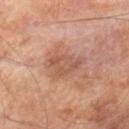workup — imaged on a skin check; not biopsied | acquisition — 15 mm crop, total-body photography | patient — male, in their mid- to late 60s | location — the right lower leg.